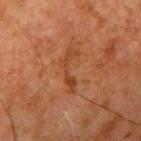No biopsy was performed on this lesion — it was imaged during a full skin examination and was not determined to be concerning.